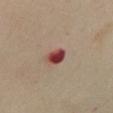| key | value |
|---|---|
| biopsy status | total-body-photography surveillance lesion; no biopsy |
| illumination | cross-polarized illumination |
| patient | female, in their mid-50s |
| image-analysis metrics | an area of roughly 4.5 mm², an outline eccentricity of about 0.55 (0 = round, 1 = elongated), and a shape-asymmetry score of about 0.2 (0 = symmetric); a lesion color around L≈44 a*≈29 b*≈24 in CIELAB and a lesion-to-skin contrast of about 12.5 (normalized; higher = more distinct); border irregularity of about 2 on a 0–10 scale, a within-lesion color-variation index near 5.5/10, and radial color variation of about 1.5 |
| location | the abdomen |
| lesion size | ≈2.5 mm |
| acquisition | total-body-photography crop, ~15 mm field of view |